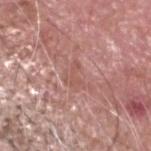biopsy_status: not biopsied; imaged during a skin examination
image:
  source: total-body photography crop
  field_of_view_mm: 15
patient:
  sex: male
  age_approx: 75
site: head or neck